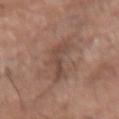Q: How large is the lesion?
A: ≈6.5 mm
Q: How was this image acquired?
A: ~15 mm tile from a whole-body skin photo
Q: How was the tile lit?
A: white-light
Q: What did automated image analysis measure?
A: a shape eccentricity near 0.95 and a shape-asymmetry score of about 0.5 (0 = symmetric); a border-irregularity index near 7/10 and peripheral color asymmetry of about 0.5; a nevus-likeness score of about 0/100 and lesion-presence confidence of about 85/100
Q: Where on the body is the lesion?
A: the right upper arm
Q: What are the patient's age and sex?
A: male, roughly 75 years of age